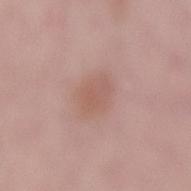{"biopsy_status": "not biopsied; imaged during a skin examination", "lighting": "white-light", "lesion_size": {"long_diameter_mm_approx": 3.0}, "site": "lower back", "patient": {"sex": "male", "age_approx": 55}, "image": {"source": "total-body photography crop", "field_of_view_mm": 15}}